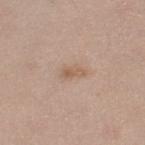The lesion was tiled from a total-body skin photograph and was not biopsied. The subject is a female about 40 years old. Imaged with white-light lighting. The lesion-visualizer software estimated a mean CIELAB color near L≈58 a*≈17 b*≈30 and a lesion-to-skin contrast of about 6 (normalized; higher = more distinct). The analysis additionally found an automated nevus-likeness rating near 10 out of 100. The lesion is on the right lower leg. A region of skin cropped from a whole-body photographic capture, roughly 15 mm wide.